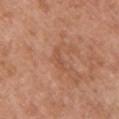Q: Was this lesion biopsied?
A: total-body-photography surveillance lesion; no biopsy
Q: What did automated image analysis measure?
A: an area of roughly 2.5 mm², an outline eccentricity of about 0.95 (0 = round, 1 = elongated), and two-axis asymmetry of about 0.35; a mean CIELAB color near L≈52 a*≈24 b*≈33, about 6 CIELAB-L* units darker than the surrounding skin, and a normalized border contrast of about 4.5; a border-irregularity index near 4/10, internal color variation of about 0 on a 0–10 scale, and peripheral color asymmetry of about 0; a classifier nevus-likeness of about 0/100 and a lesion-detection confidence of about 90/100
Q: What lighting was used for the tile?
A: white-light illumination
Q: Patient demographics?
A: female, roughly 60 years of age
Q: What is the lesion's diameter?
A: about 3 mm
Q: How was this image acquired?
A: total-body-photography crop, ~15 mm field of view
Q: Where on the body is the lesion?
A: the chest